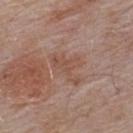Captured during whole-body skin photography for melanoma surveillance; the lesion was not biopsied. A roughly 15 mm field-of-view crop from a total-body skin photograph. On the back. A male subject, aged 53–57. Longest diameter approximately 5 mm. The total-body-photography lesion software estimated a lesion area of about 9.5 mm², a shape eccentricity near 0.75, and a symmetry-axis asymmetry near 0.5. The analysis additionally found a lesion color around L≈51 a*≈20 b*≈26 in CIELAB, about 7 CIELAB-L* units darker than the surrounding skin, and a normalized border contrast of about 5.5. The analysis additionally found an automated nevus-likeness rating near 0 out of 100 and a detector confidence of about 100 out of 100 that the crop contains a lesion.